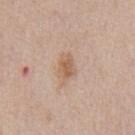Impression:
Part of a total-body skin-imaging series; this lesion was reviewed on a skin check and was not flagged for biopsy.
Background:
The lesion is located on the chest. A male subject aged approximately 60. Imaged with white-light lighting. The lesion's longest dimension is about 3 mm. A lesion tile, about 15 mm wide, cut from a 3D total-body photograph.